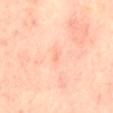No biopsy was performed on this lesion — it was imaged during a full skin examination and was not determined to be concerning. The lesion is located on the mid back. The total-body-photography lesion software estimated a mean CIELAB color near L≈76 a*≈27 b*≈34 and a normalized lesion–skin contrast near 3.5. Longest diameter approximately 1 mm. This image is a 15 mm lesion crop taken from a total-body photograph. The subject is a female approximately 40 years of age. The tile uses cross-polarized illumination.